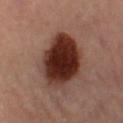Impression:
Recorded during total-body skin imaging; not selected for excision or biopsy.
Context:
Approximately 6 mm at its widest. The tile uses cross-polarized illumination. Cropped from a total-body skin-imaging series; the visible field is about 15 mm. Located on the right leg. The subject is a female aged 58 to 62.